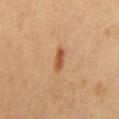The lesion was photographed on a routine skin check and not biopsied; there is no pathology result. A 15 mm crop from a total-body photograph taken for skin-cancer surveillance. The lesion is located on the front of the torso. Imaged with cross-polarized lighting. The lesion-visualizer software estimated a mean CIELAB color near L≈45 a*≈21 b*≈34, roughly 10 lightness units darker than nearby skin, and a lesion-to-skin contrast of about 8.5 (normalized; higher = more distinct). The software also gave internal color variation of about 1 on a 0–10 scale and a peripheral color-asymmetry measure near 0.5. It also reported a nevus-likeness score of about 95/100 and a detector confidence of about 100 out of 100 that the crop contains a lesion. The subject is a female about 40 years old.